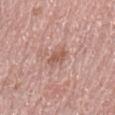follow-up: total-body-photography surveillance lesion; no biopsy
tile lighting: white-light illumination
automated metrics: a footprint of about 3.5 mm² and an outline eccentricity of about 0.7 (0 = round, 1 = elongated); a lesion color around L≈56 a*≈22 b*≈27 in CIELAB, roughly 9 lightness units darker than nearby skin, and a normalized border contrast of about 6.5; a within-lesion color-variation index near 1.5/10 and radial color variation of about 0.5; a classifier nevus-likeness of about 0/100
diameter: about 2.5 mm
subject: female, in their mid-60s
location: the left upper arm
image: 15 mm crop, total-body photography